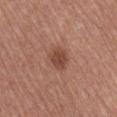Part of a total-body skin-imaging series; this lesion was reviewed on a skin check and was not flagged for biopsy. About 3 mm across. This image is a 15 mm lesion crop taken from a total-body photograph. The lesion is located on the right upper arm. Captured under white-light illumination. A male subject approximately 65 years of age.